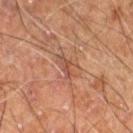| field | value |
|---|---|
| workup | no biopsy performed (imaged during a skin exam) |
| patient | male, aged around 60 |
| lesion diameter | about 2.5 mm |
| location | the right thigh |
| imaging modality | 15 mm crop, total-body photography |